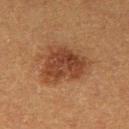<case>
  <lesion_size>
    <long_diameter_mm_approx>6.0</long_diameter_mm_approx>
  </lesion_size>
  <image>
    <source>total-body photography crop</source>
    <field_of_view_mm>15</field_of_view_mm>
  </image>
  <patient>
    <sex>female</sex>
    <age_approx>40</age_approx>
  </patient>
  <site>right thigh</site>
  <lighting>cross-polarized</lighting>
</case>